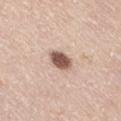patient = female, aged approximately 45 | image source = total-body-photography crop, ~15 mm field of view | anatomic site = the right thigh.